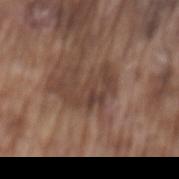Assessment:
The lesion was photographed on a routine skin check and not biopsied; there is no pathology result.